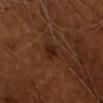Clinical impression: No biopsy was performed on this lesion — it was imaged during a full skin examination and was not determined to be concerning.